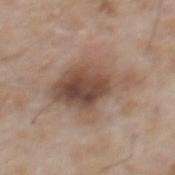follow-up — total-body-photography surveillance lesion; no biopsy
patient — male, aged around 50
image source — total-body-photography crop, ~15 mm field of view
location — the mid back